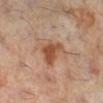The lesion was photographed on a routine skin check and not biopsied; there is no pathology result. A roughly 15 mm field-of-view crop from a total-body skin photograph. The lesion is on the right lower leg. The total-body-photography lesion software estimated a lesion area of about 8 mm² and a shape-asymmetry score of about 0.4 (0 = symmetric). And it measured a lesion color around L≈52 a*≈23 b*≈34 in CIELAB, about 11 CIELAB-L* units darker than the surrounding skin, and a lesion-to-skin contrast of about 9 (normalized; higher = more distinct). And it measured an automated nevus-likeness rating near 80 out of 100 and lesion-presence confidence of about 100/100. This is a cross-polarized tile. A female patient, aged 58–62. About 4 mm across.